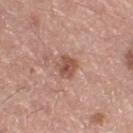Impression: No biopsy was performed on this lesion — it was imaged during a full skin examination and was not determined to be concerning.